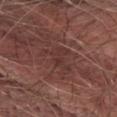follow-up = imaged on a skin check; not biopsied | body site = the abdomen | subject = male, aged approximately 75 | image = total-body-photography crop, ~15 mm field of view | diameter = ≈4.5 mm.